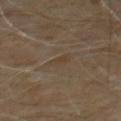* biopsy status · catalogued during a skin exam; not biopsied
* acquisition · total-body-photography crop, ~15 mm field of view
* subject · male, aged approximately 60
* body site · the front of the torso